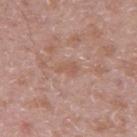anatomic site: the right upper arm | patient: male, roughly 50 years of age | size: ~2.5 mm (longest diameter) | automated lesion analysis: an outline eccentricity of about 0.8 (0 = round, 1 = elongated) and a shape-asymmetry score of about 0.4 (0 = symmetric); a mean CIELAB color near L≈56 a*≈21 b*≈27, about 6 CIELAB-L* units darker than the surrounding skin, and a normalized border contrast of about 5; a border-irregularity rating of about 4/10; an automated nevus-likeness rating near 0 out of 100 | image: ~15 mm crop, total-body skin-cancer survey.